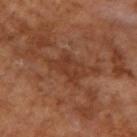Part of a total-body skin-imaging series; this lesion was reviewed on a skin check and was not flagged for biopsy. The lesion is on the arm. Cropped from a whole-body photographic skin survey; the tile spans about 15 mm. Imaged with cross-polarized lighting. The subject is a male roughly 55 years of age. The lesion's longest dimension is about 4 mm.